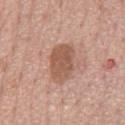Part of a total-body skin-imaging series; this lesion was reviewed on a skin check and was not flagged for biopsy.